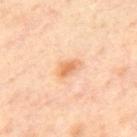workup = total-body-photography surveillance lesion; no biopsy | image source = ~15 mm crop, total-body skin-cancer survey | patient = male, aged around 45 | body site = the upper back.